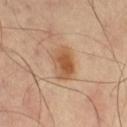| feature | finding |
|---|---|
| follow-up | catalogued during a skin exam; not biopsied |
| image | 15 mm crop, total-body photography |
| body site | the right thigh |
| illumination | cross-polarized |
| TBP lesion metrics | an automated nevus-likeness rating near 95 out of 100 and a lesion-detection confidence of about 100/100 |